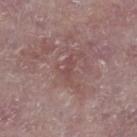| feature | finding |
|---|---|
| workup | no biopsy performed (imaged during a skin exam) |
| patient | male, approximately 75 years of age |
| site | the right lower leg |
| lighting | white-light |
| image source | total-body-photography crop, ~15 mm field of view |
| lesion size | ~2.5 mm (longest diameter) |
| TBP lesion metrics | a lesion color around L≈46 a*≈21 b*≈19 in CIELAB, roughly 6 lightness units darker than nearby skin, and a normalized border contrast of about 4.5; a within-lesion color-variation index near 0/10 |